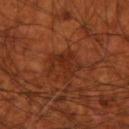Assessment: Recorded during total-body skin imaging; not selected for excision or biopsy. Image and clinical context: Cropped from a total-body skin-imaging series; the visible field is about 15 mm. A male patient, roughly 70 years of age. From the right upper arm. The recorded lesion diameter is about 4 mm.